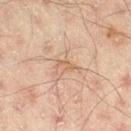<tbp_lesion>
<biopsy_status>not biopsied; imaged during a skin examination</biopsy_status>
<site>leg</site>
<automated_metrics>
  <area_mm2_approx>3.0</area_mm2_approx>
  <eccentricity>0.85</eccentricity>
  <shape_asymmetry>0.45</shape_asymmetry>
  <nevus_likeness_0_100>0</nevus_likeness_0_100>
  <lesion_detection_confidence_0_100>100</lesion_detection_confidence_0_100>
</automated_metrics>
<lighting>cross-polarized</lighting>
<image>
  <source>total-body photography crop</source>
  <field_of_view_mm>15</field_of_view_mm>
</image>
<patient>
  <sex>male</sex>
  <age_approx>45</age_approx>
</patient>
<lesion_size>
  <long_diameter_mm_approx>2.5</long_diameter_mm_approx>
</lesion_size>
</tbp_lesion>